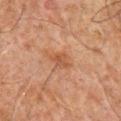Clinical summary: Imaged with cross-polarized lighting. The lesion is located on the chest. The subject is a male about 60 years old. Cropped from a total-body skin-imaging series; the visible field is about 15 mm. Longest diameter approximately 3 mm. The total-body-photography lesion software estimated two-axis asymmetry of about 0.2. The analysis additionally found an automated nevus-likeness rating near 0 out of 100 and lesion-presence confidence of about 100/100.